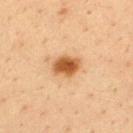The lesion is located on the upper back.
A male subject, roughly 35 years of age.
Cropped from a whole-body photographic skin survey; the tile spans about 15 mm.
This is a cross-polarized tile.
The recorded lesion diameter is about 3.5 mm.